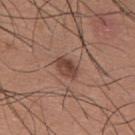This lesion was catalogued during total-body skin photography and was not selected for biopsy. A male subject aged 33–37. This image is a 15 mm lesion crop taken from a total-body photograph. Located on the upper back.